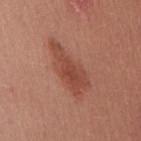No biopsy was performed on this lesion — it was imaged during a full skin examination and was not determined to be concerning.